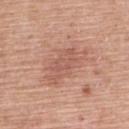The lesion-visualizer software estimated an average lesion color of about L≈57 a*≈23 b*≈28 (CIELAB), roughly 7 lightness units darker than nearby skin, and a normalized border contrast of about 5. It also reported a nevus-likeness score of about 0/100. The lesion's longest dimension is about 5.5 mm. The lesion is on the upper back. The subject is a male roughly 60 years of age. Cropped from a total-body skin-imaging series; the visible field is about 15 mm.